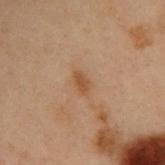Captured during whole-body skin photography for melanoma surveillance; the lesion was not biopsied. The subject is a male aged 53 to 57. A region of skin cropped from a whole-body photographic capture, roughly 15 mm wide. From the right upper arm. Longest diameter approximately 2.5 mm. Imaged with cross-polarized lighting. Automated tile analysis of the lesion measured a footprint of about 3 mm², an eccentricity of roughly 0.85, and a symmetry-axis asymmetry near 0.25. The analysis additionally found a lesion color around L≈40 a*≈17 b*≈29 in CIELAB, roughly 7 lightness units darker than nearby skin, and a normalized border contrast of about 6.5. It also reported an automated nevus-likeness rating near 15 out of 100 and lesion-presence confidence of about 100/100.